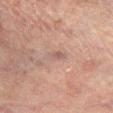<lesion>
<biopsy_status>not biopsied; imaged during a skin examination</biopsy_status>
<automated_metrics>
  <nevus_likeness_0_100>0</nevus_likeness_0_100>
</automated_metrics>
<site>leg</site>
<lesion_size>
  <long_diameter_mm_approx>2.5</long_diameter_mm_approx>
</lesion_size>
<image>
  <source>total-body photography crop</source>
  <field_of_view_mm>15</field_of_view_mm>
</image>
<lighting>cross-polarized</lighting>
<patient>
  <sex>male</sex>
  <age_approx>75</age_approx>
</patient>
</lesion>